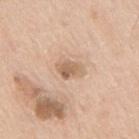Impression:
The lesion was tiled from a total-body skin photograph and was not biopsied.
Acquisition and patient details:
A close-up tile cropped from a whole-body skin photograph, about 15 mm across. A male patient aged around 65. Located on the mid back.